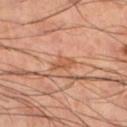automated metrics = an area of roughly 3.5 mm², a shape eccentricity near 0.85, and two-axis asymmetry of about 0.25; a mean CIELAB color near L≈56 a*≈25 b*≈35 and a lesion–skin lightness drop of about 8; a within-lesion color-variation index near 1.5/10 and peripheral color asymmetry of about 0.5; a classifier nevus-likeness of about 0/100 and a detector confidence of about 100 out of 100 that the crop contains a lesion | tile lighting = cross-polarized illumination | patient = male, roughly 50 years of age | acquisition = ~15 mm tile from a whole-body skin photo | size = about 3 mm | site = the left lower leg.